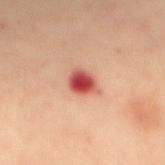site = the back
illumination = cross-polarized illumination
patient = female, aged around 45
acquisition = 15 mm crop, total-body photography
lesion size = ~3 mm (longest diameter)
automated lesion analysis = a footprint of about 5.5 mm², a shape eccentricity near 0.5, and a symmetry-axis asymmetry near 0.2; a border-irregularity rating of about 1.5/10, a within-lesion color-variation index near 6.5/10, and radial color variation of about 2; a classifier nevus-likeness of about 0/100 and a lesion-detection confidence of about 100/100